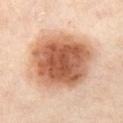Captured during whole-body skin photography for melanoma surveillance; the lesion was not biopsied.
The patient is a female aged 53 to 57.
A 15 mm crop from a total-body photograph taken for skin-cancer surveillance.
Longest diameter approximately 8.5 mm.
Imaged with cross-polarized lighting.
On the left thigh.
The total-body-photography lesion software estimated a lesion color around L≈59 a*≈23 b*≈33 in CIELAB, about 18 CIELAB-L* units darker than the surrounding skin, and a lesion-to-skin contrast of about 11 (normalized; higher = more distinct). The software also gave border irregularity of about 2 on a 0–10 scale, internal color variation of about 7 on a 0–10 scale, and a peripheral color-asymmetry measure near 1.5.